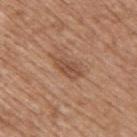No biopsy was performed on this lesion — it was imaged during a full skin examination and was not determined to be concerning. Imaged with white-light lighting. A male patient, aged approximately 60. Located on the upper back. This image is a 15 mm lesion crop taken from a total-body photograph. An algorithmic analysis of the crop reported a mean CIELAB color near L≈50 a*≈21 b*≈31, about 9 CIELAB-L* units darker than the surrounding skin, and a normalized lesion–skin contrast near 6.5. And it measured a border-irregularity rating of about 3/10 and a peripheral color-asymmetry measure near 1.